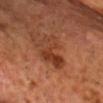<record>
<biopsy_status>not biopsied; imaged during a skin examination</biopsy_status>
<lesion_size>
  <long_diameter_mm_approx>5.5</long_diameter_mm_approx>
</lesion_size>
<patient>
  <sex>male</sex>
  <age_approx>70</age_approx>
</patient>
<site>chest</site>
<image>
  <source>total-body photography crop</source>
  <field_of_view_mm>15</field_of_view_mm>
</image>
<automated_metrics>
  <area_mm2_approx>11.0</area_mm2_approx>
  <eccentricity>0.9</eccentricity>
  <vs_skin_darker_L>9.0</vs_skin_darker_L>
  <vs_skin_contrast_norm>7.0</vs_skin_contrast_norm>
  <border_irregularity_0_10>6.5</border_irregularity_0_10>
  <color_variation_0_10>7.0</color_variation_0_10>
  <peripheral_color_asymmetry>2.0</peripheral_color_asymmetry>
  <nevus_likeness_0_100>60</nevus_likeness_0_100>
  <lesion_detection_confidence_0_100>100</lesion_detection_confidence_0_100>
</automated_metrics>
<lighting>cross-polarized</lighting>
</record>